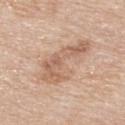| key | value |
|---|---|
| notes | total-body-photography surveillance lesion; no biopsy |
| imaging modality | total-body-photography crop, ~15 mm field of view |
| diameter | ~7 mm (longest diameter) |
| TBP lesion metrics | border irregularity of about 7 on a 0–10 scale, internal color variation of about 3.5 on a 0–10 scale, and radial color variation of about 1; a nevus-likeness score of about 0/100 and a lesion-detection confidence of about 100/100 |
| location | the upper back |
| patient | male, aged around 85 or older |
| lighting | white-light illumination |